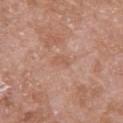The tile uses white-light illumination. The lesion-visualizer software estimated two-axis asymmetry of about 0.4. And it measured roughly 6 lightness units darker than nearby skin and a normalized border contrast of about 4.5. It also reported border irregularity of about 3.5 on a 0–10 scale. It also reported a lesion-detection confidence of about 100/100. The lesion is on the arm. Cropped from a whole-body photographic skin survey; the tile spans about 15 mm. A female patient, approximately 70 years of age.